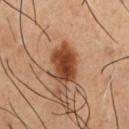| feature | finding |
|---|---|
| notes | catalogued during a skin exam; not biopsied |
| subject | male, approximately 55 years of age |
| image source | total-body-photography crop, ~15 mm field of view |
| anatomic site | the chest |